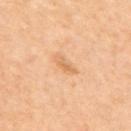Assessment:
Part of a total-body skin-imaging series; this lesion was reviewed on a skin check and was not flagged for biopsy.
Clinical summary:
The total-body-photography lesion software estimated a footprint of about 2.5 mm². The software also gave a mean CIELAB color near L≈65 a*≈22 b*≈40. The analysis additionally found a border-irregularity rating of about 4/10, internal color variation of about 0 on a 0–10 scale, and peripheral color asymmetry of about 0. This is a cross-polarized tile. The patient is a female about 50 years old. A region of skin cropped from a whole-body photographic capture, roughly 15 mm wide. About 2.5 mm across. From the upper back.